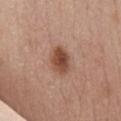follow-up = catalogued during a skin exam; not biopsied | lighting = white-light illumination | size = ~3.5 mm (longest diameter) | patient = female, aged 43 to 47 | imaging modality = total-body-photography crop, ~15 mm field of view | location = the chest.